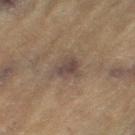Recorded during total-body skin imaging; not selected for excision or biopsy. Cropped from a total-body skin-imaging series; the visible field is about 15 mm. Imaged with cross-polarized lighting. The patient is a female aged approximately 80. The lesion is located on the left thigh.